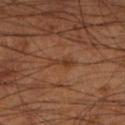- notes · imaged on a skin check; not biopsied
- TBP lesion metrics · a classifier nevus-likeness of about 0/100 and a lesion-detection confidence of about 90/100
- subject · male, aged around 55
- image · 15 mm crop, total-body photography
- body site · the left lower leg
- lesion size · ~3 mm (longest diameter)
- illumination · cross-polarized